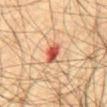Assessment:
Recorded during total-body skin imaging; not selected for excision or biopsy.
Background:
A male subject, aged around 65. The tile uses cross-polarized illumination. On the front of the torso. A 15 mm close-up tile from a total-body photography series done for melanoma screening. Longest diameter approximately 3 mm.